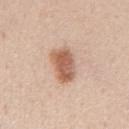Part of a total-body skin-imaging series; this lesion was reviewed on a skin check and was not flagged for biopsy. From the mid back. The total-body-photography lesion software estimated an area of roughly 10 mm², an outline eccentricity of about 0.85 (0 = round, 1 = elongated), and a shape-asymmetry score of about 0.2 (0 = symmetric). And it measured an average lesion color of about L≈60 a*≈22 b*≈31 (CIELAB) and roughly 14 lightness units darker than nearby skin. The software also gave a border-irregularity index near 2.5/10, a color-variation rating of about 4.5/10, and radial color variation of about 1.5. The recorded lesion diameter is about 4.5 mm. A 15 mm close-up tile from a total-body photography series done for melanoma screening. A male patient approximately 50 years of age.